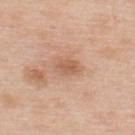Notes:
* subject: male, in their 50s
* body site: the upper back
* acquisition: 15 mm crop, total-body photography